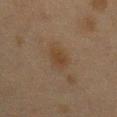Cropped from a whole-body photographic skin survey; the tile spans about 15 mm. A female subject aged approximately 40. The total-body-photography lesion software estimated an area of roughly 4 mm² and an eccentricity of roughly 0.65. And it measured a border-irregularity rating of about 2/10, a within-lesion color-variation index near 1.5/10, and a peripheral color-asymmetry measure near 0.5. On the chest.